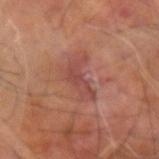Imaged during a routine full-body skin examination; the lesion was not biopsied and no histopathology is available.
A 15 mm crop from a total-body photograph taken for skin-cancer surveillance.
On the right arm.
A male subject, aged 58 to 62.